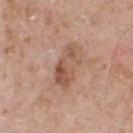workup — no biopsy performed (imaged during a skin exam)
illumination — white-light
subject — male, in their mid- to late 50s
acquisition — ~15 mm crop, total-body skin-cancer survey
body site — the upper back
automated metrics — an area of roughly 10 mm², an outline eccentricity of about 0.9 (0 = round, 1 = elongated), and a symmetry-axis asymmetry near 0.3; an automated nevus-likeness rating near 0 out of 100
size — ~5 mm (longest diameter)